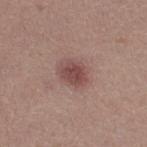Q: Was a biopsy performed?
A: catalogued during a skin exam; not biopsied
Q: How was this image acquired?
A: total-body-photography crop, ~15 mm field of view
Q: Where on the body is the lesion?
A: the right thigh
Q: Lesion size?
A: ~3.5 mm (longest diameter)
Q: Who is the patient?
A: female, aged approximately 20